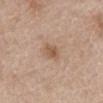Captured during whole-body skin photography for melanoma surveillance; the lesion was not biopsied.
A region of skin cropped from a whole-body photographic capture, roughly 15 mm wide.
The lesion is on the abdomen.
The recorded lesion diameter is about 3 mm.
A female patient, in their mid-60s.
Captured under white-light illumination.
Automated image analysis of the tile measured about 9 CIELAB-L* units darker than the surrounding skin and a normalized border contrast of about 6.5. It also reported a border-irregularity index near 2/10, a within-lesion color-variation index near 3/10, and peripheral color asymmetry of about 1.